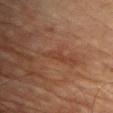Q: Was a biopsy performed?
A: catalogued during a skin exam; not biopsied
Q: What is the lesion's diameter?
A: about 3 mm
Q: What is the anatomic site?
A: the front of the torso
Q: What are the patient's age and sex?
A: male, approximately 75 years of age
Q: Illumination type?
A: cross-polarized illumination
Q: Automated lesion metrics?
A: an average lesion color of about L≈34 a*≈20 b*≈26 (CIELAB), a lesion–skin lightness drop of about 4, and a normalized border contrast of about 4.5; a border-irregularity index near 4.5/10, a within-lesion color-variation index near 0/10, and peripheral color asymmetry of about 0; a detector confidence of about 65 out of 100 that the crop contains a lesion
Q: How was this image acquired?
A: ~15 mm crop, total-body skin-cancer survey